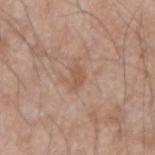Assessment:
Recorded during total-body skin imaging; not selected for excision or biopsy.
Context:
A male patient, aged 68 to 72. This is a white-light tile. Measured at roughly 2.5 mm in maximum diameter. Cropped from a total-body skin-imaging series; the visible field is about 15 mm. Located on the right forearm. An algorithmic analysis of the crop reported a lesion color around L≈55 a*≈19 b*≈30 in CIELAB and a normalized lesion–skin contrast near 5.5. It also reported a border-irregularity index near 4/10, a within-lesion color-variation index near 0.5/10, and radial color variation of about 0. The analysis additionally found an automated nevus-likeness rating near 0 out of 100 and a detector confidence of about 100 out of 100 that the crop contains a lesion.